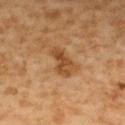Findings:
* notes · no biopsy performed (imaged during a skin exam)
* patient · male, in their 60s
* acquisition · 15 mm crop, total-body photography
* lighting · cross-polarized
* site · the back